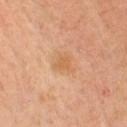The lesion was tiled from a total-body skin photograph and was not biopsied. The patient is a female aged 38 to 42. From the chest. Automated tile analysis of the lesion measured an eccentricity of roughly 0.4 and a symmetry-axis asymmetry near 0.4. And it measured a lesion–skin lightness drop of about 6. The analysis additionally found a border-irregularity rating of about 4/10, a color-variation rating of about 1/10, and peripheral color asymmetry of about 0.5. A close-up tile cropped from a whole-body skin photograph, about 15 mm across.